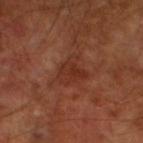Clinical impression:
The lesion was tiled from a total-body skin photograph and was not biopsied.
Clinical summary:
Cropped from a whole-body photographic skin survey; the tile spans about 15 mm. Located on the left lower leg. Automated tile analysis of the lesion measured a border-irregularity index near 4.5/10, a within-lesion color-variation index near 3/10, and radial color variation of about 1. The analysis additionally found a classifier nevus-likeness of about 0/100 and lesion-presence confidence of about 100/100. This is a cross-polarized tile. The subject is a male aged 58 to 62.